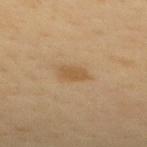Acquisition and patient details:
The patient is a female aged around 50. The lesion is located on the upper back. Imaged with cross-polarized lighting. A close-up tile cropped from a whole-body skin photograph, about 15 mm across.